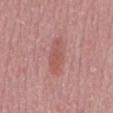– notes: no biopsy performed (imaged during a skin exam)
– illumination: white-light
– automated lesion analysis: an automated nevus-likeness rating near 15 out of 100 and a detector confidence of about 100 out of 100 that the crop contains a lesion
– subject: male, approximately 50 years of age
– image source: total-body-photography crop, ~15 mm field of view
– anatomic site: the mid back